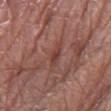Q: What are the patient's age and sex?
A: male, aged 78 to 82
Q: How was the tile lit?
A: white-light
Q: Lesion location?
A: the right thigh
Q: What kind of image is this?
A: 15 mm crop, total-body photography